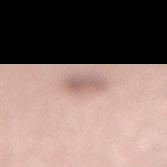The lesion was photographed on a routine skin check and not biopsied; there is no pathology result. This is a white-light tile. From the right lower leg. The total-body-photography lesion software estimated a lesion area of about 5 mm², an eccentricity of roughly 0.75, and a shape-asymmetry score of about 0.25 (0 = symmetric). The software also gave an average lesion color of about L≈62 a*≈18 b*≈22 (CIELAB), roughly 11 lightness units darker than nearby skin, and a normalized border contrast of about 7. And it measured border irregularity of about 2 on a 0–10 scale, internal color variation of about 3 on a 0–10 scale, and radial color variation of about 1. Longest diameter approximately 3 mm. A female subject in their mid-40s. A 15 mm crop from a total-body photograph taken for skin-cancer surveillance.